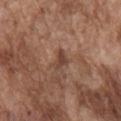Imaged during a routine full-body skin examination; the lesion was not biopsied and no histopathology is available.
Approximately 2.5 mm at its widest.
A close-up tile cropped from a whole-body skin photograph, about 15 mm across.
On the chest.
Captured under white-light illumination.
A male subject aged 73–77.